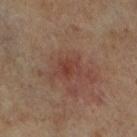Captured during whole-body skin photography for melanoma surveillance; the lesion was not biopsied. This image is a 15 mm lesion crop taken from a total-body photograph. Longest diameter approximately 2.5 mm. Imaged with cross-polarized lighting. A female patient approximately 65 years of age. On the left leg.